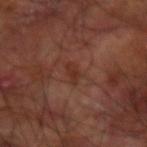Imaged during a routine full-body skin examination; the lesion was not biopsied and no histopathology is available.
A male patient aged approximately 60.
A 15 mm crop from a total-body photograph taken for skin-cancer surveillance.
The tile uses cross-polarized illumination.
Approximately 2.5 mm at its widest.
Located on the right arm.